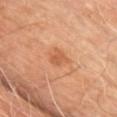The lesion was tiled from a total-body skin photograph and was not biopsied.
The total-body-photography lesion software estimated a footprint of about 3 mm², an outline eccentricity of about 0.55 (0 = round, 1 = elongated), and two-axis asymmetry of about 0.2. And it measured a classifier nevus-likeness of about 85/100.
The patient is a male approximately 50 years of age.
On the chest.
A roughly 15 mm field-of-view crop from a total-body skin photograph.
This is a cross-polarized tile.
About 2 mm across.